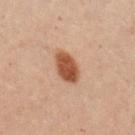Assessment: Part of a total-body skin-imaging series; this lesion was reviewed on a skin check and was not flagged for biopsy. Background: The lesion is located on the right upper arm. This image is a 15 mm lesion crop taken from a total-body photograph. A male patient, aged 28 to 32. Automated tile analysis of the lesion measured a mean CIELAB color near L≈41 a*≈20 b*≈29, about 13 CIELAB-L* units darker than the surrounding skin, and a normalized border contrast of about 11. It also reported a color-variation rating of about 3/10 and a peripheral color-asymmetry measure near 1. Measured at roughly 4 mm in maximum diameter. Captured under cross-polarized illumination.